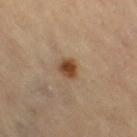Background: The patient is a female aged around 70. An algorithmic analysis of the crop reported a classifier nevus-likeness of about 100/100 and a lesion-detection confidence of about 100/100. Captured under cross-polarized illumination. A roughly 15 mm field-of-view crop from a total-body skin photograph. On the leg.